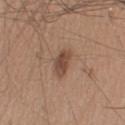This is a white-light tile. A 15 mm close-up tile from a total-body photography series done for melanoma screening. The recorded lesion diameter is about 3.5 mm. Located on the left upper arm. A male patient, aged 53–57.